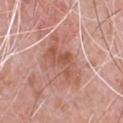Impression:
No biopsy was performed on this lesion — it was imaged during a full skin examination and was not determined to be concerning.
Acquisition and patient details:
From the chest. A 15 mm crop from a total-body photograph taken for skin-cancer surveillance. The lesion's longest dimension is about 6.5 mm. A male patient aged approximately 50. Captured under white-light illumination.